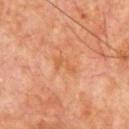Case summary:
* biopsy status — imaged on a skin check; not biopsied